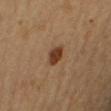{"biopsy_status": "not biopsied; imaged during a skin examination", "image": {"source": "total-body photography crop", "field_of_view_mm": 15}, "automated_metrics": {"area_mm2_approx": 4.5, "shape_asymmetry": 0.15, "cielab_L": 34, "cielab_a": 19, "cielab_b": 29, "vs_skin_darker_L": 12.0, "vs_skin_contrast_norm": 10.5, "border_irregularity_0_10": 1.0, "color_variation_0_10": 2.5, "peripheral_color_asymmetry": 1.0, "nevus_likeness_0_100": 100, "lesion_detection_confidence_0_100": 100}, "site": "right upper arm", "lighting": "cross-polarized", "lesion_size": {"long_diameter_mm_approx": 2.5}, "patient": {"sex": "male", "age_approx": 60}}